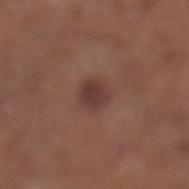<case>
<biopsy_status>not biopsied; imaged during a skin examination</biopsy_status>
<image>
  <source>total-body photography crop</source>
  <field_of_view_mm>15</field_of_view_mm>
</image>
<lesion_size>
  <long_diameter_mm_approx>3.0</long_diameter_mm_approx>
</lesion_size>
<patient>
  <sex>male</sex>
  <age_approx>70</age_approx>
</patient>
<site>leg</site>
<lighting>white-light</lighting>
</case>